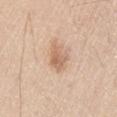The lesion was photographed on a routine skin check and not biopsied; there is no pathology result. The tile uses white-light illumination. A male subject, in their 60s. This image is a 15 mm lesion crop taken from a total-body photograph. About 3.5 mm across. Located on the leg.